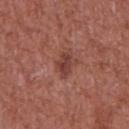The lesion was photographed on a routine skin check and not biopsied; there is no pathology result.
This image is a 15 mm lesion crop taken from a total-body photograph.
The lesion is on the chest.
A male patient aged 63 to 67.
Longest diameter approximately 3 mm.
The tile uses white-light illumination.